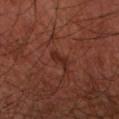* biopsy status — no biopsy performed (imaged during a skin exam)
* illumination — cross-polarized illumination
* lesion size — about 2.5 mm
* patient — male, about 65 years old
* imaging modality — ~15 mm tile from a whole-body skin photo
* location — the left forearm
* TBP lesion metrics — a footprint of about 2.5 mm², a shape eccentricity near 0.9, and two-axis asymmetry of about 0.35; a lesion color around L≈26 a*≈24 b*≈26 in CIELAB and a lesion-to-skin contrast of about 7 (normalized; higher = more distinct); a within-lesion color-variation index near 0/10 and a peripheral color-asymmetry measure near 0